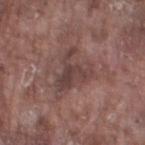biopsy_status: not biopsied; imaged during a skin examination
patient:
  sex: male
  age_approx: 75
automated_metrics:
  vs_skin_darker_L: 8.0
  vs_skin_contrast_norm: 7.0
site: left thigh
lesion_size:
  long_diameter_mm_approx: 5.0
lighting: white-light
image:
  source: total-body photography crop
  field_of_view_mm: 15